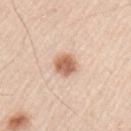This lesion was catalogued during total-body skin photography and was not selected for biopsy. This image is a 15 mm lesion crop taken from a total-body photograph. The lesion is on the arm. Measured at roughly 3 mm in maximum diameter. This is a white-light tile. The subject is a male aged approximately 45. Automated image analysis of the tile measured about 15 CIELAB-L* units darker than the surrounding skin and a normalized border contrast of about 9. The software also gave an automated nevus-likeness rating near 100 out of 100 and a lesion-detection confidence of about 100/100.